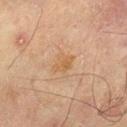workup: no biopsy performed (imaged during a skin exam); diameter: ~2.5 mm (longest diameter); lighting: cross-polarized; automated lesion analysis: a shape-asymmetry score of about 0.25 (0 = symmetric); image: total-body-photography crop, ~15 mm field of view; site: the left thigh; patient: male, in their 70s.